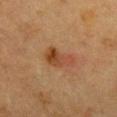Q: Was this lesion biopsied?
A: no biopsy performed (imaged during a skin exam)
Q: Where on the body is the lesion?
A: the chest
Q: How was this image acquired?
A: ~15 mm tile from a whole-body skin photo
Q: What are the patient's age and sex?
A: female, in their mid- to late 50s